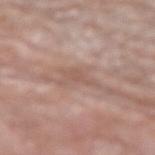Clinical summary: The lesion-visualizer software estimated border irregularity of about 4.5 on a 0–10 scale and radial color variation of about 0.5. And it measured a nevus-likeness score of about 0/100 and lesion-presence confidence of about 60/100. Located on the right forearm. The lesion's longest dimension is about 3 mm. Captured under white-light illumination. Cropped from a total-body skin-imaging series; the visible field is about 15 mm. A male subject, roughly 80 years of age.